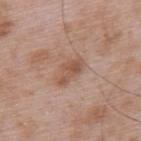Impression: The lesion was photographed on a routine skin check and not biopsied; there is no pathology result. Clinical summary: A male subject, in their 50s. On the back. A lesion tile, about 15 mm wide, cut from a 3D total-body photograph. The total-body-photography lesion software estimated an outline eccentricity of about 0.9 (0 = round, 1 = elongated) and two-axis asymmetry of about 0.4. It also reported a lesion color around L≈53 a*≈20 b*≈29 in CIELAB, roughly 9 lightness units darker than nearby skin, and a normalized border contrast of about 7. And it measured a nevus-likeness score of about 10/100 and a detector confidence of about 100 out of 100 that the crop contains a lesion.